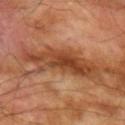Acquisition and patient details:
Automated tile analysis of the lesion measured a lesion area of about 20 mm², an outline eccentricity of about 0.95 (0 = round, 1 = elongated), and two-axis asymmetry of about 0.35. The software also gave internal color variation of about 5.5 on a 0–10 scale and radial color variation of about 2. The analysis additionally found a detector confidence of about 90 out of 100 that the crop contains a lesion. Cropped from a whole-body photographic skin survey; the tile spans about 15 mm. The recorded lesion diameter is about 8.5 mm. The lesion is located on the arm. A male patient roughly 70 years of age.